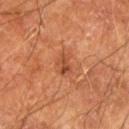follow-up: no biopsy performed (imaged during a skin exam) | size: about 3 mm | site: the arm | TBP lesion metrics: a lesion color around L≈47 a*≈27 b*≈36 in CIELAB, a lesion–skin lightness drop of about 9, and a lesion-to-skin contrast of about 6.5 (normalized; higher = more distinct); a border-irregularity index near 3.5/10 | patient: male, aged 63–67 | image source: 15 mm crop, total-body photography.